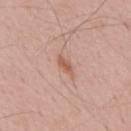A region of skin cropped from a whole-body photographic capture, roughly 15 mm wide.
A male patient in their mid- to late 50s.